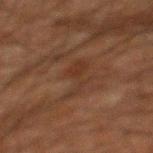| key | value |
|---|---|
| follow-up | catalogued during a skin exam; not biopsied |
| imaging modality | ~15 mm tile from a whole-body skin photo |
| subject | male, aged around 60 |
| lesion size | ≈5 mm |
| site | the mid back |